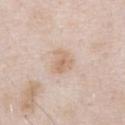Case summary:
- biopsy status: no biopsy performed (imaged during a skin exam)
- image-analysis metrics: an outline eccentricity of about 0.65 (0 = round, 1 = elongated) and a symmetry-axis asymmetry near 0.3; a lesion–skin lightness drop of about 8 and a normalized lesion–skin contrast near 6; border irregularity of about 2.5 on a 0–10 scale and a within-lesion color-variation index near 3/10; a nevus-likeness score of about 5/100
- imaging modality: 15 mm crop, total-body photography
- patient: male, in their mid- to late 50s
- lighting: white-light illumination
- anatomic site: the chest
- lesion diameter: about 3 mm